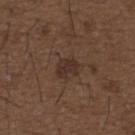- workup — no biopsy performed (imaged during a skin exam)
- location — the back
- subject — male, about 50 years old
- acquisition — ~15 mm tile from a whole-body skin photo
- diameter — ≈3 mm